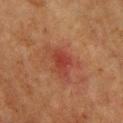| key | value |
|---|---|
| follow-up | total-body-photography surveillance lesion; no biopsy |
| illumination | cross-polarized |
| acquisition | total-body-photography crop, ~15 mm field of view |
| lesion diameter | about 3 mm |
| patient | male, aged approximately 60 |
| body site | the chest |